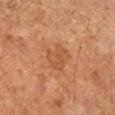biopsy_status: not biopsied; imaged during a skin examination